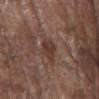lesion_size:
  long_diameter_mm_approx: 3.5
lighting: white-light
image:
  source: total-body photography crop
  field_of_view_mm: 15
patient:
  sex: male
  age_approx: 80
site: chest